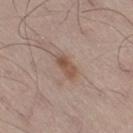Captured during whole-body skin photography for melanoma surveillance; the lesion was not biopsied.